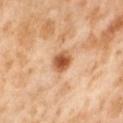follow-up: no biopsy performed (imaged during a skin exam)
acquisition: total-body-photography crop, ~15 mm field of view
patient: female, aged 53 to 57
TBP lesion metrics: a footprint of about 5.5 mm², an outline eccentricity of about 0.3 (0 = round, 1 = elongated), and two-axis asymmetry of about 0.2; a mean CIELAB color near L≈58 a*≈25 b*≈39 and a lesion-to-skin contrast of about 10 (normalized; higher = more distinct); border irregularity of about 1.5 on a 0–10 scale and a color-variation rating of about 4/10; a nevus-likeness score of about 95/100 and lesion-presence confidence of about 100/100
body site: the right thigh
lesion size: about 2.5 mm